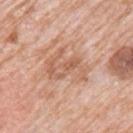{"biopsy_status": "not biopsied; imaged during a skin examination", "automated_metrics": {"vs_skin_darker_L": 9.0, "vs_skin_contrast_norm": 6.0, "border_irregularity_0_10": 5.0, "color_variation_0_10": 5.0, "peripheral_color_asymmetry": 1.5, "nevus_likeness_0_100": 0}, "lesion_size": {"long_diameter_mm_approx": 5.0}, "lighting": "white-light", "patient": {"sex": "male", "age_approx": 80}, "image": {"source": "total-body photography crop", "field_of_view_mm": 15}, "site": "left upper arm"}